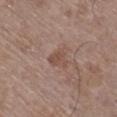Q: Was this lesion biopsied?
A: catalogued during a skin exam; not biopsied
Q: What is the imaging modality?
A: ~15 mm tile from a whole-body skin photo
Q: Who is the patient?
A: male, in their mid-60s
Q: What is the anatomic site?
A: the right lower leg
Q: How was the tile lit?
A: white-light illumination
Q: Automated lesion metrics?
A: an area of roughly 4 mm² and a shape-asymmetry score of about 0.4 (0 = symmetric); a mean CIELAB color near L≈49 a*≈18 b*≈24 and a lesion-to-skin contrast of about 5.5 (normalized; higher = more distinct); a classifier nevus-likeness of about 0/100 and lesion-presence confidence of about 100/100